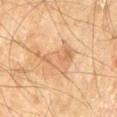  biopsy_status: not biopsied; imaged during a skin examination
  site: left thigh
  automated_metrics:
    area_mm2_approx: 10.0
    eccentricity: 0.85
    shape_asymmetry: 0.5
    cielab_L: 65
    cielab_a: 21
    cielab_b: 38
    vs_skin_darker_L: 8.0
    vs_skin_contrast_norm: 5.0
    nevus_likeness_0_100: 0
    lesion_detection_confidence_0_100: 100
  lighting: cross-polarized
  lesion_size:
    long_diameter_mm_approx: 5.5
  patient:
    sex: male
    age_approx: 70
  image:
    source: total-body photography crop
    field_of_view_mm: 15